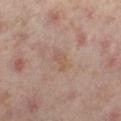The lesion was tiled from a total-body skin photograph and was not biopsied. Located on the leg. The subject is a female roughly 50 years of age. This is a cross-polarized tile. Measured at roughly 3 mm in maximum diameter. Cropped from a whole-body photographic skin survey; the tile spans about 15 mm.